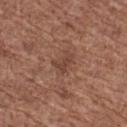• automated metrics: about 7 CIELAB-L* units darker than the surrounding skin and a normalized border contrast of about 6; a border-irregularity index near 5.5/10, internal color variation of about 0 on a 0–10 scale, and radial color variation of about 0
• diameter: about 3 mm
• subject: female, aged 73–77
• image: ~15 mm tile from a whole-body skin photo
• location: the right thigh
• lighting: white-light illumination